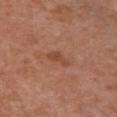A 15 mm close-up tile from a total-body photography series done for melanoma screening.
The patient is a female aged approximately 80.
Imaged with white-light lighting.
Located on the chest.
Approximately 3 mm at its widest.
Automated tile analysis of the lesion measured a lesion area of about 3 mm² and two-axis asymmetry of about 0.4. The analysis additionally found a border-irregularity rating of about 4.5/10 and peripheral color asymmetry of about 0. And it measured an automated nevus-likeness rating near 0 out of 100 and a lesion-detection confidence of about 100/100.